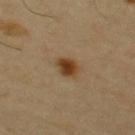biopsy status: no biopsy performed (imaged during a skin exam) | anatomic site: the back | image source: 15 mm crop, total-body photography | patient: male, aged approximately 65 | lesion size: ≈3 mm | illumination: cross-polarized illumination.